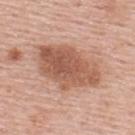Clinical impression:
Part of a total-body skin-imaging series; this lesion was reviewed on a skin check and was not flagged for biopsy.
Clinical summary:
The tile uses white-light illumination. Located on the upper back. Cropped from a whole-body photographic skin survey; the tile spans about 15 mm. A male subject, in their mid-50s.